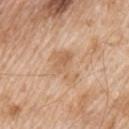The patient is a male in their mid-60s.
About 4.5 mm across.
Automated tile analysis of the lesion measured an average lesion color of about L≈62 a*≈19 b*≈35 (CIELAB), about 8 CIELAB-L* units darker than the surrounding skin, and a lesion-to-skin contrast of about 5.5 (normalized; higher = more distinct). It also reported peripheral color asymmetry of about 1. The software also gave a classifier nevus-likeness of about 0/100 and lesion-presence confidence of about 100/100.
A 15 mm crop from a total-body photograph taken for skin-cancer surveillance.
Captured under white-light illumination.
On the left upper arm.